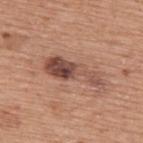Impression:
This lesion was catalogued during total-body skin photography and was not selected for biopsy.
Context:
A male subject approximately 75 years of age. On the upper back. The lesion's longest dimension is about 7 mm. The tile uses white-light illumination. The lesion-visualizer software estimated a lesion area of about 12 mm², an eccentricity of roughly 0.95, and a shape-asymmetry score of about 0.45 (0 = symmetric). Cropped from a total-body skin-imaging series; the visible field is about 15 mm.